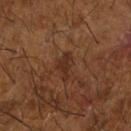Impression:
This lesion was catalogued during total-body skin photography and was not selected for biopsy.
Clinical summary:
From the right forearm. A male patient, aged approximately 65. Imaged with cross-polarized lighting. Cropped from a total-body skin-imaging series; the visible field is about 15 mm.